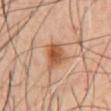Impression:
No biopsy was performed on this lesion — it was imaged during a full skin examination and was not determined to be concerning.
Image and clinical context:
The patient is a male aged 48–52. The lesion is on the chest. A lesion tile, about 15 mm wide, cut from a 3D total-body photograph.